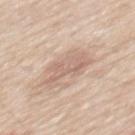workup: total-body-photography surveillance lesion; no biopsy | patient: male, aged approximately 60 | lesion diameter: ~5 mm (longest diameter) | image source: total-body-photography crop, ~15 mm field of view | anatomic site: the mid back | image-analysis metrics: an area of roughly 11 mm² and a shape eccentricity near 0.8; a lesion color around L≈65 a*≈17 b*≈26 in CIELAB, roughly 8 lightness units darker than nearby skin, and a lesion-to-skin contrast of about 5.5 (normalized; higher = more distinct); border irregularity of about 5 on a 0–10 scale and peripheral color asymmetry of about 1; an automated nevus-likeness rating near 0 out of 100 | tile lighting: white-light illumination.